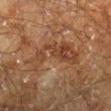Assessment: This lesion was catalogued during total-body skin photography and was not selected for biopsy. Clinical summary: The lesion is on the right lower leg. A lesion tile, about 15 mm wide, cut from a 3D total-body photograph. Imaged with cross-polarized lighting. A male patient, approximately 60 years of age. Automated image analysis of the tile measured about 6 CIELAB-L* units darker than the surrounding skin and a normalized lesion–skin contrast near 5.5. And it measured a border-irregularity index near 9.5/10, a color-variation rating of about 4/10, and radial color variation of about 1. The analysis additionally found a nevus-likeness score of about 0/100 and lesion-presence confidence of about 65/100.